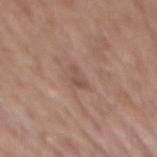Captured under white-light illumination. A female subject, in their mid- to late 60s. From the back. Cropped from a total-body skin-imaging series; the visible field is about 15 mm.